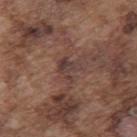Impression: The lesion was photographed on a routine skin check and not biopsied; there is no pathology result. Image and clinical context: The lesion-visualizer software estimated an eccentricity of roughly 0.5. And it measured a lesion color around L≈38 a*≈17 b*≈20 in CIELAB, about 7 CIELAB-L* units darker than the surrounding skin, and a normalized lesion–skin contrast near 7.5. The software also gave internal color variation of about 3 on a 0–10 scale and peripheral color asymmetry of about 1. The software also gave a classifier nevus-likeness of about 0/100 and a lesion-detection confidence of about 60/100. The subject is a male aged 73–77. Captured under white-light illumination. From the mid back. Cropped from a total-body skin-imaging series; the visible field is about 15 mm.